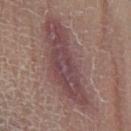Clinical impression:
The lesion was photographed on a routine skin check and not biopsied; there is no pathology result.
Background:
The patient is a male aged 83 or older. The total-body-photography lesion software estimated a lesion color around L≈43 a*≈18 b*≈17 in CIELAB, a lesion–skin lightness drop of about 9, and a normalized border contrast of about 8. It also reported an automated nevus-likeness rating near 0 out of 100 and a detector confidence of about 75 out of 100 that the crop contains a lesion. Captured under cross-polarized illumination. From the leg. A roughly 15 mm field-of-view crop from a total-body skin photograph.